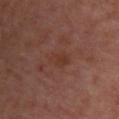| field | value |
|---|---|
| tile lighting | cross-polarized |
| location | the chest |
| subject | aged 53–57 |
| image source | ~15 mm crop, total-body skin-cancer survey |